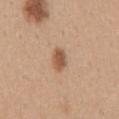image: ~15 mm crop, total-body skin-cancer survey
body site: the mid back
diameter: ~3 mm (longest diameter)
patient: female, aged 28 to 32
image-analysis metrics: an average lesion color of about L≈55 a*≈20 b*≈32 (CIELAB) and roughly 12 lightness units darker than nearby skin; a border-irregularity index near 1.5/10, a color-variation rating of about 2.5/10, and a peripheral color-asymmetry measure near 1